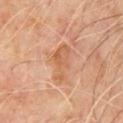biopsy_status: not biopsied; imaged during a skin examination
site: chest
image:
  source: total-body photography crop
  field_of_view_mm: 15
patient:
  sex: male
  age_approx: 70
lesion_size:
  long_diameter_mm_approx: 5.0
lighting: cross-polarized